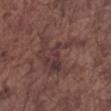Impression: Part of a total-body skin-imaging series; this lesion was reviewed on a skin check and was not flagged for biopsy. Clinical summary: Cropped from a whole-body photographic skin survey; the tile spans about 15 mm. From the right forearm. A male subject roughly 75 years of age.